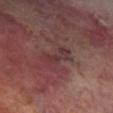biopsy_status: not biopsied; imaged during a skin examination
lighting: cross-polarized
patient:
  sex: male
  age_approx: 70
lesion_size:
  long_diameter_mm_approx: 4.5
site: right lower leg
image:
  source: total-body photography crop
  field_of_view_mm: 15